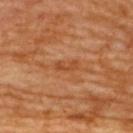Assessment: Part of a total-body skin-imaging series; this lesion was reviewed on a skin check and was not flagged for biopsy. Image and clinical context: Imaged with cross-polarized lighting. The lesion is located on the upper back. The total-body-photography lesion software estimated a lesion–skin lightness drop of about 7 and a normalized border contrast of about 6.5. The analysis additionally found a nevus-likeness score of about 0/100 and a detector confidence of about 100 out of 100 that the crop contains a lesion. A female subject, aged approximately 65. The lesion's longest dimension is about 3 mm. A roughly 15 mm field-of-view crop from a total-body skin photograph.